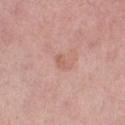Captured during whole-body skin photography for melanoma surveillance; the lesion was not biopsied. From the right thigh. A region of skin cropped from a whole-body photographic capture, roughly 15 mm wide. A female patient, aged 48–52. Automated tile analysis of the lesion measured an outline eccentricity of about 0.85 (0 = round, 1 = elongated) and a shape-asymmetry score of about 0.35 (0 = symmetric). This is a white-light tile.